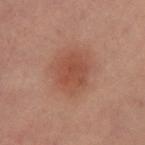A female patient approximately 40 years of age.
Imaged with cross-polarized lighting.
About 4.5 mm across.
A region of skin cropped from a whole-body photographic capture, roughly 15 mm wide.
From the right thigh.